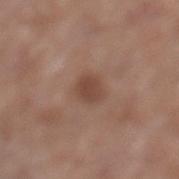Q: Was a biopsy performed?
A: catalogued during a skin exam; not biopsied
Q: What is the anatomic site?
A: the leg
Q: Who is the patient?
A: female, in their mid- to late 70s
Q: What kind of image is this?
A: total-body-photography crop, ~15 mm field of view